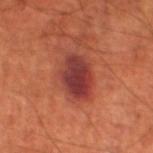workup: catalogued during a skin exam; not biopsied
lesion diameter: ~5 mm (longest diameter)
subject: male, aged around 70
TBP lesion metrics: a lesion area of about 14 mm², an outline eccentricity of about 0.75 (0 = round, 1 = elongated), and a symmetry-axis asymmetry near 0.15; a mean CIELAB color near L≈39 a*≈30 b*≈27, roughly 12 lightness units darker than nearby skin, and a normalized lesion–skin contrast near 11
site: the leg
tile lighting: cross-polarized illumination
image source: total-body-photography crop, ~15 mm field of view